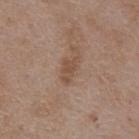  biopsy_status: not biopsied; imaged during a skin examination
  image:
    source: total-body photography crop
    field_of_view_mm: 15
  site: back
  lesion_size:
    long_diameter_mm_approx: 3.0
  patient:
    sex: female
    age_approx: 70